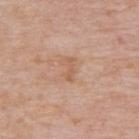The lesion was photographed on a routine skin check and not biopsied; there is no pathology result.
A 15 mm close-up extracted from a 3D total-body photography capture.
Approximately 2.5 mm at its widest.
On the upper back.
An algorithmic analysis of the crop reported an average lesion color of about L≈59 a*≈21 b*≈32 (CIELAB), about 7 CIELAB-L* units darker than the surrounding skin, and a normalized border contrast of about 5.5. The software also gave a nevus-likeness score of about 0/100.
This is a white-light tile.
The patient is a male approximately 75 years of age.